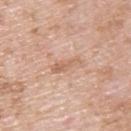Findings:
- biopsy status — no biopsy performed (imaged during a skin exam)
- location — the upper back
- image source — ~15 mm crop, total-body skin-cancer survey
- image-analysis metrics — a border-irregularity index near 4.5/10, a color-variation rating of about 1/10, and a peripheral color-asymmetry measure near 0
- subject — male, about 50 years old
- lighting — white-light illumination